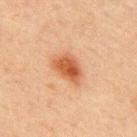Imaged during a routine full-body skin examination; the lesion was not biopsied and no histopathology is available.